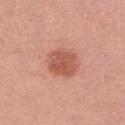<case>
<biopsy_status>not biopsied; imaged during a skin examination</biopsy_status>
<lesion_size>
  <long_diameter_mm_approx>4.0</long_diameter_mm_approx>
</lesion_size>
<lighting>white-light</lighting>
<image>
  <source>total-body photography crop</source>
  <field_of_view_mm>15</field_of_view_mm>
</image>
<site>arm</site>
<patient>
  <sex>female</sex>
  <age_approx>35</age_approx>
</patient>
</case>